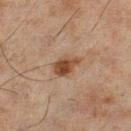Case summary:
– notes — no biopsy performed (imaged during a skin exam)
– lesion size — about 3.5 mm
– subject — male, approximately 45 years of age
– image source — total-body-photography crop, ~15 mm field of view
– site — the left lower leg
– illumination — cross-polarized